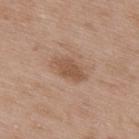No biopsy was performed on this lesion — it was imaged during a full skin examination and was not determined to be concerning.
A close-up tile cropped from a whole-body skin photograph, about 15 mm across.
The lesion is located on the upper back.
Automated image analysis of the tile measured a mean CIELAB color near L≈53 a*≈19 b*≈30, roughly 9 lightness units darker than nearby skin, and a normalized border contrast of about 7. And it measured border irregularity of about 2.5 on a 0–10 scale, internal color variation of about 2.5 on a 0–10 scale, and a peripheral color-asymmetry measure near 1.
The subject is a male aged 48 to 52.
Captured under white-light illumination.